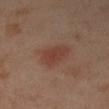Imaged during a routine full-body skin examination; the lesion was not biopsied and no histopathology is available.
The patient is a female aged approximately 40.
Imaged with cross-polarized lighting.
Located on the left arm.
A close-up tile cropped from a whole-body skin photograph, about 15 mm across.